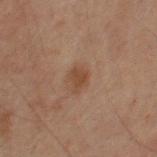Part of a total-body skin-imaging series; this lesion was reviewed on a skin check and was not flagged for biopsy. From the arm. This is a cross-polarized tile. The recorded lesion diameter is about 3 mm. Cropped from a whole-body photographic skin survey; the tile spans about 15 mm. The patient is a male about 70 years old.